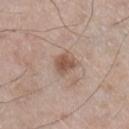Assessment:
This lesion was catalogued during total-body skin photography and was not selected for biopsy.
Background:
The lesion is on the leg. Approximately 3 mm at its widest. Captured under white-light illumination. A roughly 15 mm field-of-view crop from a total-body skin photograph. The subject is a male aged 58 to 62.